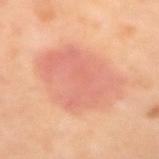The lesion was tiled from a total-body skin photograph and was not biopsied.